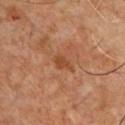Clinical impression: Captured during whole-body skin photography for melanoma surveillance; the lesion was not biopsied. Clinical summary: Automated tile analysis of the lesion measured a footprint of about 4 mm² and an eccentricity of roughly 0.8. A region of skin cropped from a whole-body photographic capture, roughly 15 mm wide. A male subject in their 50s. Imaged with cross-polarized lighting. The lesion's longest dimension is about 3 mm. From the chest.